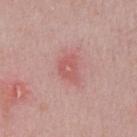Notes:
- biopsy status: no biopsy performed (imaged during a skin exam)
- anatomic site: the chest
- imaging modality: ~15 mm tile from a whole-body skin photo
- tile lighting: white-light
- patient: male, aged 48 to 52
- lesion size: ~3 mm (longest diameter)